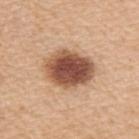| feature | finding |
|---|---|
| workup | no biopsy performed (imaged during a skin exam) |